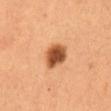Captured during whole-body skin photography for melanoma surveillance; the lesion was not biopsied. On the abdomen. A female subject. Cropped from a whole-body photographic skin survey; the tile spans about 15 mm.